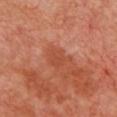A female subject approximately 50 years of age. On the chest. A 15 mm close-up tile from a total-body photography series done for melanoma screening.